The lesion is located on the abdomen · a lesion tile, about 15 mm wide, cut from a 3D total-body photograph · a female patient, aged 23 to 27 · the recorded lesion diameter is about 7 mm · An algorithmic analysis of the crop reported an area of roughly 30 mm², an eccentricity of roughly 0.65, and a shape-asymmetry score of about 0.1 (0 = symmetric). The analysis additionally found an average lesion color of about L≈57 a*≈27 b*≈38 (CIELAB), about 24 CIELAB-L* units darker than the surrounding skin, and a normalized border contrast of about 14. And it measured a border-irregularity index near 1/10, internal color variation of about 8.5 on a 0–10 scale, and peripheral color asymmetry of about 2.5. And it measured a nevus-likeness score of about 100/100 and lesion-presence confidence of about 100/100 · the tile uses cross-polarized illumination — 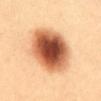– diagnosis — an atypical melanocytic neoplasm (borderline)